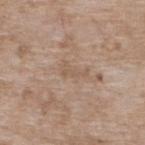Notes:
• follow-up: catalogued during a skin exam; not biopsied
• anatomic site: the upper back
• subject: female, approximately 75 years of age
• diameter: ≈3 mm
• acquisition: ~15 mm tile from a whole-body skin photo
• tile lighting: white-light